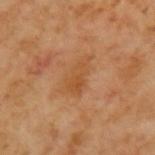| field | value |
|---|---|
| follow-up | no biopsy performed (imaged during a skin exam) |